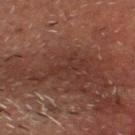lesion size = ≈4 mm | image source = ~15 mm tile from a whole-body skin photo | body site = the head or neck | patient = male, aged approximately 60 | lighting = cross-polarized.